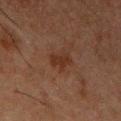workup: no biopsy performed (imaged during a skin exam) | acquisition: 15 mm crop, total-body photography | size: ~3 mm (longest diameter) | anatomic site: the chest | illumination: cross-polarized illumination | subject: male, aged around 50 | image-analysis metrics: a shape eccentricity near 0.6 and two-axis asymmetry of about 0.3; a lesion color around L≈24 a*≈17 b*≈22 in CIELAB, a lesion–skin lightness drop of about 5, and a lesion-to-skin contrast of about 6.5 (normalized; higher = more distinct); a border-irregularity rating of about 3.5/10, a within-lesion color-variation index near 2/10, and a peripheral color-asymmetry measure near 0.5; a nevus-likeness score of about 55/100.